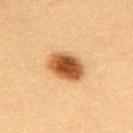<tbp_lesion>
  <biopsy_status>not biopsied; imaged during a skin examination</biopsy_status>
  <image>
    <source>total-body photography crop</source>
    <field_of_view_mm>15</field_of_view_mm>
  </image>
  <site>upper back</site>
  <patient>
    <sex>female</sex>
    <age_approx>30</age_approx>
  </patient>
</tbp_lesion>